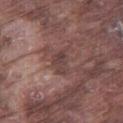biopsy status = catalogued during a skin exam; not biopsied | illumination = white-light | subject = male, roughly 75 years of age | image source = ~15 mm crop, total-body skin-cancer survey | body site = the leg | diameter = ~3.5 mm (longest diameter).